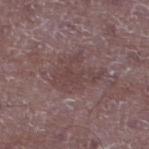Recorded during total-body skin imaging; not selected for excision or biopsy.
The tile uses white-light illumination.
A close-up tile cropped from a whole-body skin photograph, about 15 mm across.
Automated image analysis of the tile measured a shape eccentricity near 0.7 and a symmetry-axis asymmetry near 0.6. The software also gave an average lesion color of about L≈42 a*≈18 b*≈17 (CIELAB), about 6 CIELAB-L* units darker than the surrounding skin, and a normalized border contrast of about 5.5. It also reported border irregularity of about 9 on a 0–10 scale, internal color variation of about 2 on a 0–10 scale, and a peripheral color-asymmetry measure near 0.5. The analysis additionally found lesion-presence confidence of about 65/100.
The subject is a male aged approximately 50.
About 5.5 mm across.
From the left lower leg.